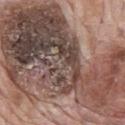workup = catalogued during a skin exam; not biopsied | imaging modality = ~15 mm tile from a whole-body skin photo | illumination = white-light | patient = male, roughly 70 years of age | location = the mid back | size = ≈22 mm.